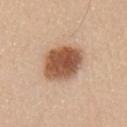follow-up: total-body-photography surveillance lesion; no biopsy | body site: the chest | diameter: about 4.5 mm | image: ~15 mm tile from a whole-body skin photo | tile lighting: white-light | subject: male, aged 38 to 42.